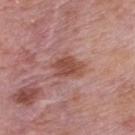follow-up — no biopsy performed (imaged during a skin exam); subject — male, aged 73–77; acquisition — ~15 mm crop, total-body skin-cancer survey; diameter — ≈4 mm; location — the mid back; tile lighting — white-light illumination.